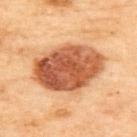| key | value |
|---|---|
| body site | the upper back |
| automated lesion analysis | a footprint of about 33 mm², an eccentricity of roughly 0.7, and a shape-asymmetry score of about 0.1 (0 = symmetric); an average lesion color of about L≈57 a*≈28 b*≈39 (CIELAB), about 19 CIELAB-L* units darker than the surrounding skin, and a lesion-to-skin contrast of about 11.5 (normalized; higher = more distinct); a nevus-likeness score of about 80/100 and a detector confidence of about 100 out of 100 that the crop contains a lesion |
| image source | ~15 mm tile from a whole-body skin photo |
| subject | female, aged approximately 60 |
| illumination | cross-polarized illumination |
| lesion diameter | ~8 mm (longest diameter) |